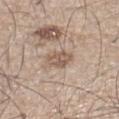This lesion was catalogued during total-body skin photography and was not selected for biopsy. Cropped from a total-body skin-imaging series; the visible field is about 15 mm. The lesion is on the leg. The patient is a male about 45 years old. The lesion-visualizer software estimated border irregularity of about 2.5 on a 0–10 scale, internal color variation of about 3 on a 0–10 scale, and peripheral color asymmetry of about 1.